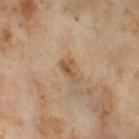Part of a total-body skin-imaging series; this lesion was reviewed on a skin check and was not flagged for biopsy. Located on the leg. A female patient, aged approximately 55. A 15 mm close-up tile from a total-body photography series done for melanoma screening.